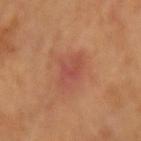{
  "biopsy_status": "not biopsied; imaged during a skin examination",
  "lesion_size": {
    "long_diameter_mm_approx": 3.5
  },
  "site": "arm",
  "patient": {
    "age_approx": 60
  },
  "image": {
    "source": "total-body photography crop",
    "field_of_view_mm": 15
  }
}